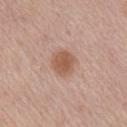biopsy_status: not biopsied; imaged during a skin examination
site: right upper arm
patient:
  sex: male
  age_approx: 65
lighting: white-light
automated_metrics:
  area_mm2_approx: 7.5
  eccentricity: 0.4
  shape_asymmetry: 0.15
  border_irregularity_0_10: 1.5
image:
  source: total-body photography crop
  field_of_view_mm: 15